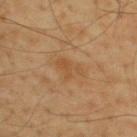Recorded during total-body skin imaging; not selected for excision or biopsy. Imaged with cross-polarized lighting. From the upper back. Approximately 3.5 mm at its widest. A 15 mm crop from a total-body photograph taken for skin-cancer surveillance. A male patient, in their mid- to late 50s.